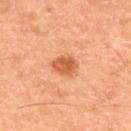Assessment:
Captured during whole-body skin photography for melanoma surveillance; the lesion was not biopsied.
Acquisition and patient details:
Measured at roughly 3 mm in maximum diameter. The patient is a male aged 58–62. A region of skin cropped from a whole-body photographic capture, roughly 15 mm wide. Imaged with cross-polarized lighting. An algorithmic analysis of the crop reported two-axis asymmetry of about 0.2.